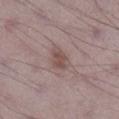follow-up: no biopsy performed (imaged during a skin exam); image source: ~15 mm crop, total-body skin-cancer survey; diameter: about 3 mm; patient: male, aged 68–72; location: the right lower leg; tile lighting: white-light illumination.